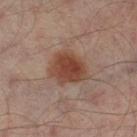The lesion was tiled from a total-body skin photograph and was not biopsied. Captured under cross-polarized illumination. A region of skin cropped from a whole-body photographic capture, roughly 15 mm wide. A male subject approximately 65 years of age. Located on the left thigh. The recorded lesion diameter is about 5 mm.